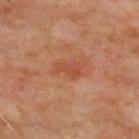Captured during whole-body skin photography for melanoma surveillance; the lesion was not biopsied. Located on the upper back. A 15 mm close-up tile from a total-body photography series done for melanoma screening. A male subject in their mid-60s.